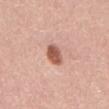The lesion was tiled from a total-body skin photograph and was not biopsied. Cropped from a total-body skin-imaging series; the visible field is about 15 mm. Imaged with white-light lighting. The subject is a male in their mid- to late 40s. On the abdomen.